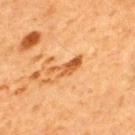A male subject aged around 65.
Cropped from a total-body skin-imaging series; the visible field is about 15 mm.
Imaged with cross-polarized lighting.
Located on the back.
Measured at roughly 4.5 mm in maximum diameter.